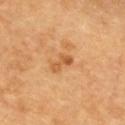The patient is in their 60s.
Imaged with cross-polarized lighting.
The lesion is located on the upper back.
Longest diameter approximately 3 mm.
A close-up tile cropped from a whole-body skin photograph, about 15 mm across.